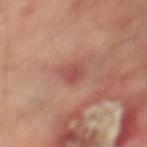location: the left thigh
image: total-body-photography crop, ~15 mm field of view
patient: male, in their mid- to late 70s
lighting: cross-polarized illumination